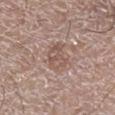biopsy status = catalogued during a skin exam; not biopsied | site = the leg | acquisition = total-body-photography crop, ~15 mm field of view | lesion size = ~4 mm (longest diameter) | lighting = white-light illumination | automated lesion analysis = a mean CIELAB color near L≈54 a*≈17 b*≈24 and a lesion–skin lightness drop of about 7; a border-irregularity rating of about 3/10 and a within-lesion color-variation index near 3.5/10 | subject = male, aged 58–62.